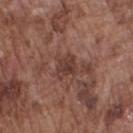Clinical impression: Captured during whole-body skin photography for melanoma surveillance; the lesion was not biopsied. Background: An algorithmic analysis of the crop reported a border-irregularity rating of about 3.5/10, a color-variation rating of about 3/10, and a peripheral color-asymmetry measure near 1. A male patient, aged approximately 75. From the right upper arm. Measured at roughly 3 mm in maximum diameter. A roughly 15 mm field-of-view crop from a total-body skin photograph. This is a white-light tile.